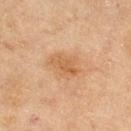biopsy status = catalogued during a skin exam; not biopsied
lighting = cross-polarized
lesion size = about 4 mm
subject = female, in their 60s
image = ~15 mm crop, total-body skin-cancer survey
location = the right thigh